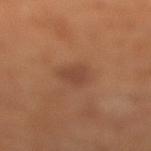Recorded during total-body skin imaging; not selected for excision or biopsy.
A female subject in their mid-60s.
A lesion tile, about 15 mm wide, cut from a 3D total-body photograph.
Longest diameter approximately 2.5 mm.
From the right lower leg.
This is a cross-polarized tile.